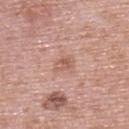About 2.5 mm across. The lesion is on the upper back. Imaged with white-light lighting. A 15 mm close-up tile from a total-body photography series done for melanoma screening. The patient is a female roughly 60 years of age.